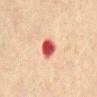Q: Is there a histopathology result?
A: no biopsy performed (imaged during a skin exam)
Q: Lesion location?
A: the mid back
Q: Who is the patient?
A: male, in their 70s
Q: Lesion size?
A: ~2.5 mm (longest diameter)
Q: What did automated image analysis measure?
A: a lesion area of about 5 mm², an eccentricity of roughly 0.5, and a symmetry-axis asymmetry near 0.25; a lesion color around L≈45 a*≈34 b*≈27 in CIELAB, a lesion–skin lightness drop of about 19, and a normalized border contrast of about 13
Q: What lighting was used for the tile?
A: cross-polarized
Q: How was this image acquired?
A: total-body-photography crop, ~15 mm field of view